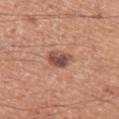The lesion was tiled from a total-body skin photograph and was not biopsied. A 15 mm close-up extracted from a 3D total-body photography capture. The subject is a male roughly 55 years of age. Approximately 3 mm at its widest. Automated tile analysis of the lesion measured an area of roughly 5.5 mm², an outline eccentricity of about 0.65 (0 = round, 1 = elongated), and a shape-asymmetry score of about 0.25 (0 = symmetric). It also reported a lesion–skin lightness drop of about 13 and a normalized lesion–skin contrast near 9.5. And it measured an automated nevus-likeness rating near 70 out of 100 and a lesion-detection confidence of about 100/100. The tile uses white-light illumination. The lesion is on the left thigh.